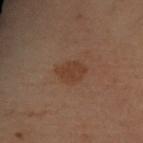A 15 mm crop from a total-body photograph taken for skin-cancer surveillance.
The tile uses cross-polarized illumination.
The patient is a female approximately 55 years of age.
Approximately 4 mm at its widest.
The lesion is located on the left arm.